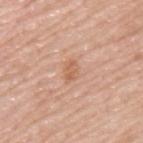biopsy status = total-body-photography surveillance lesion; no biopsy | patient = male, aged around 55 | lesion size = ≈2.5 mm | illumination = white-light illumination | image = ~15 mm tile from a whole-body skin photo | body site = the upper back | image-analysis metrics = a classifier nevus-likeness of about 0/100.